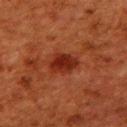Background: A close-up tile cropped from a whole-body skin photograph, about 15 mm across. The recorded lesion diameter is about 3.5 mm. A female subject roughly 50 years of age. Located on the upper back. The lesion-visualizer software estimated an area of roughly 6.5 mm², an eccentricity of roughly 0.7, and two-axis asymmetry of about 0.25. And it measured an average lesion color of about L≈24 a*≈28 b*≈28 (CIELAB), roughly 9 lightness units darker than nearby skin, and a lesion-to-skin contrast of about 9.5 (normalized; higher = more distinct). The analysis additionally found a border-irregularity index near 2/10 and a color-variation rating of about 2.5/10.